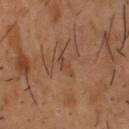workup: imaged on a skin check; not biopsied
image: total-body-photography crop, ~15 mm field of view
diameter: ~3 mm (longest diameter)
subject: male, aged around 55
lighting: cross-polarized
site: the back